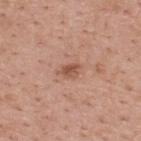The lesion was tiled from a total-body skin photograph and was not biopsied. Automated image analysis of the tile measured a shape eccentricity near 0.75 and a shape-asymmetry score of about 0.35 (0 = symmetric). The analysis additionally found a lesion color around L≈53 a*≈23 b*≈30 in CIELAB, roughly 10 lightness units darker than nearby skin, and a normalized lesion–skin contrast near 7.5. The software also gave a border-irregularity rating of about 3/10, internal color variation of about 3 on a 0–10 scale, and a peripheral color-asymmetry measure near 1. Cropped from a whole-body photographic skin survey; the tile spans about 15 mm. The tile uses white-light illumination. From the upper back. A male subject, aged around 40. The recorded lesion diameter is about 2.5 mm.